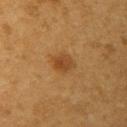Imaged during a routine full-body skin examination; the lesion was not biopsied and no histopathology is available. This image is a 15 mm lesion crop taken from a total-body photograph. The tile uses cross-polarized illumination. The lesion is located on the left upper arm. Approximately 3 mm at its widest. A male patient aged approximately 55.